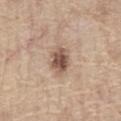| field | value |
|---|---|
| biopsy status | no biopsy performed (imaged during a skin exam) |
| patient | male, aged approximately 65 |
| tile lighting | white-light |
| location | the chest |
| image | total-body-photography crop, ~15 mm field of view |
| lesion diameter | about 3.5 mm |
| TBP lesion metrics | an area of roughly 7 mm², an outline eccentricity of about 0.75 (0 = round, 1 = elongated), and a symmetry-axis asymmetry near 0.25; an average lesion color of about L≈53 a*≈18 b*≈26 (CIELAB), a lesion–skin lightness drop of about 14, and a lesion-to-skin contrast of about 9.5 (normalized; higher = more distinct); a border-irregularity index near 2.5/10, internal color variation of about 5 on a 0–10 scale, and a peripheral color-asymmetry measure near 2; a classifier nevus-likeness of about 90/100 and lesion-presence confidence of about 100/100 |